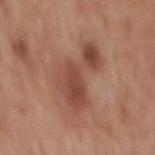– biopsy status · no biopsy performed (imaged during a skin exam)
– image · 15 mm crop, total-body photography
– image-analysis metrics · a mean CIELAB color near L≈47 a*≈23 b*≈27 and a lesion–skin lightness drop of about 10; a nevus-likeness score of about 0/100
– anatomic site · the back
– tile lighting · white-light
– patient · male, roughly 60 years of age